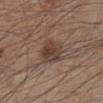follow-up = no biopsy performed (imaged during a skin exam) | acquisition = ~15 mm crop, total-body skin-cancer survey | site = the left lower leg | subject = male, aged 43–47 | lesion size = about 3.5 mm.